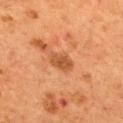<record>
<biopsy_status>not biopsied; imaged during a skin examination</biopsy_status>
<patient>
  <sex>male</sex>
  <age_approx>55</age_approx>
</patient>
<image>
  <source>total-body photography crop</source>
  <field_of_view_mm>15</field_of_view_mm>
</image>
<site>mid back</site>
<automated_metrics>
  <border_irregularity_0_10>2.5</border_irregularity_0_10>
  <color_variation_0_10>2.5</color_variation_0_10>
  <peripheral_color_asymmetry>1.0</peripheral_color_asymmetry>
  <nevus_likeness_0_100>45</nevus_likeness_0_100>
  <lesion_detection_confidence_0_100>100</lesion_detection_confidence_0_100>
</automated_metrics>
<lesion_size>
  <long_diameter_mm_approx>3.5</long_diameter_mm_approx>
</lesion_size>
<lighting>cross-polarized</lighting>
</record>